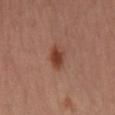Clinical impression:
Imaged during a routine full-body skin examination; the lesion was not biopsied and no histopathology is available.
Context:
A female patient approximately 40 years of age. The tile uses cross-polarized illumination. A roughly 15 mm field-of-view crop from a total-body skin photograph. Located on the left leg.